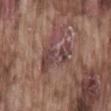Recorded during total-body skin imaging; not selected for excision or biopsy.
A close-up tile cropped from a whole-body skin photograph, about 15 mm across.
Imaged with white-light lighting.
Longest diameter approximately 5 mm.
The lesion is located on the lower back.
A male subject, in their mid- to late 70s.
An algorithmic analysis of the crop reported a lesion area of about 12 mm² and a shape-asymmetry score of about 0.55 (0 = symmetric). The analysis additionally found a nevus-likeness score of about 0/100 and lesion-presence confidence of about 75/100.